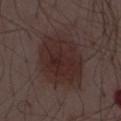biopsy_status: not biopsied; imaged during a skin examination